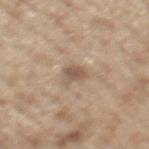Case summary:
• follow-up: no biopsy performed (imaged during a skin exam)
• subject: male, roughly 70 years of age
• TBP lesion metrics: a lesion area of about 4 mm², an outline eccentricity of about 0.75 (0 = round, 1 = elongated), and two-axis asymmetry of about 0.4; a mean CIELAB color near L≈55 a*≈14 b*≈28, a lesion–skin lightness drop of about 10, and a normalized border contrast of about 7
• diameter: ≈3 mm
• acquisition: total-body-photography crop, ~15 mm field of view
• anatomic site: the mid back
• illumination: white-light